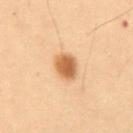Clinical impression: Imaged during a routine full-body skin examination; the lesion was not biopsied and no histopathology is available. Background: A 15 mm close-up tile from a total-body photography series done for melanoma screening. The lesion is on the abdomen. A male subject, in their mid- to late 50s. Measured at roughly 3.5 mm in maximum diameter.